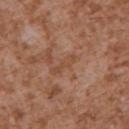biopsy_status: not biopsied; imaged during a skin examination
patient:
  sex: male
  age_approx: 45
site: arm
lighting: white-light
image:
  source: total-body photography crop
  field_of_view_mm: 15